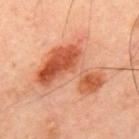| feature | finding |
|---|---|
| subject | male, aged 48 to 52 |
| body site | the upper back |
| lighting | cross-polarized |
| automated metrics | an area of roughly 25 mm² and a shape eccentricity near 0.7; a mean CIELAB color near L≈56 a*≈30 b*≈38; a lesion-detection confidence of about 100/100 |
| lesion diameter | ~7.5 mm (longest diameter) |
| image | total-body-photography crop, ~15 mm field of view |